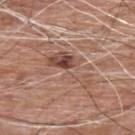Part of a total-body skin-imaging series; this lesion was reviewed on a skin check and was not flagged for biopsy.
An algorithmic analysis of the crop reported a lesion area of about 15 mm², an eccentricity of roughly 0.85, and a symmetry-axis asymmetry near 0.6.
The subject is a male roughly 60 years of age.
This image is a 15 mm lesion crop taken from a total-body photograph.
On the upper back.